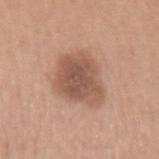follow-up: total-body-photography surveillance lesion; no biopsy
imaging modality: ~15 mm crop, total-body skin-cancer survey
lesion diameter: ~5 mm (longest diameter)
patient: male, aged 58–62
automated metrics: a lesion area of about 19 mm²
tile lighting: white-light
body site: the left upper arm